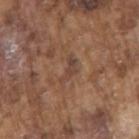No biopsy was performed on this lesion — it was imaged during a full skin examination and was not determined to be concerning. A male subject aged around 75. A 15 mm close-up extracted from a 3D total-body photography capture. From the right upper arm.